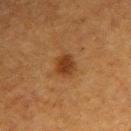Imaged during a routine full-body skin examination; the lesion was not biopsied and no histopathology is available.
Located on the right upper arm.
The recorded lesion diameter is about 2.5 mm.
A 15 mm close-up tile from a total-body photography series done for melanoma screening.
The subject is a male approximately 50 years of age.
Captured under cross-polarized illumination.
The total-body-photography lesion software estimated a lesion color around L≈30 a*≈19 b*≈31 in CIELAB, roughly 9 lightness units darker than nearby skin, and a normalized lesion–skin contrast near 9.